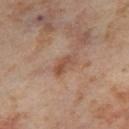{"biopsy_status": "not biopsied; imaged during a skin examination", "patient": {"sex": "female", "age_approx": 55}, "image": {"source": "total-body photography crop", "field_of_view_mm": 15}, "lesion_size": {"long_diameter_mm_approx": 3.5}, "site": "right thigh", "lighting": "cross-polarized", "automated_metrics": {"vs_skin_darker_L": 8.0, "vs_skin_contrast_norm": 6.5, "nevus_likeness_0_100": 0, "lesion_detection_confidence_0_100": 100}}